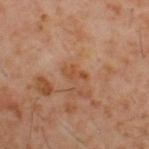Impression:
This lesion was catalogued during total-body skin photography and was not selected for biopsy.
Acquisition and patient details:
Located on the back. A 15 mm close-up extracted from a 3D total-body photography capture. A male patient, about 60 years old. Approximately 3 mm at its widest. The total-body-photography lesion software estimated a mean CIELAB color near L≈45 a*≈22 b*≈33 and a normalized border contrast of about 6. The analysis additionally found a border-irregularity index near 6/10. It also reported an automated nevus-likeness rating near 0 out of 100.